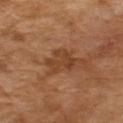workup: no biopsy performed (imaged during a skin exam); image source: 15 mm crop, total-body photography; subject: male, aged approximately 65.